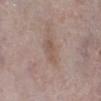Part of a total-body skin-imaging series; this lesion was reviewed on a skin check and was not flagged for biopsy.